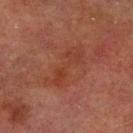Notes:
• biopsy status · catalogued during a skin exam; not biopsied
• tile lighting · cross-polarized
• automated lesion analysis · an area of roughly 11 mm², a shape eccentricity near 0.95, and a symmetry-axis asymmetry near 0.45; a border-irregularity index near 7.5/10, internal color variation of about 4 on a 0–10 scale, and radial color variation of about 1.5; a classifier nevus-likeness of about 0/100 and a detector confidence of about 100 out of 100 that the crop contains a lesion
• patient · male, about 75 years old
• size · ≈6.5 mm
• image source · ~15 mm crop, total-body skin-cancer survey
• anatomic site · the left lower leg